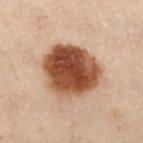biopsy status = catalogued during a skin exam; not biopsied
location = the left leg
lesion diameter = about 7 mm
image source = ~15 mm crop, total-body skin-cancer survey
patient = female, approximately 60 years of age
lighting = cross-polarized illumination